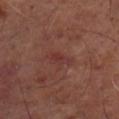Q: Was a biopsy performed?
A: total-body-photography surveillance lesion; no biopsy
Q: How was this image acquired?
A: 15 mm crop, total-body photography
Q: Automated lesion metrics?
A: a border-irregularity rating of about 4.5/10; a classifier nevus-likeness of about 0/100 and lesion-presence confidence of about 95/100
Q: Lesion location?
A: the left lower leg
Q: How large is the lesion?
A: about 3 mm
Q: Who is the patient?
A: male, in their mid- to late 60s